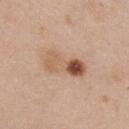Clinical impression:
The lesion was photographed on a routine skin check and not biopsied; there is no pathology result.
Image and clinical context:
A female subject aged around 45. Measured at roughly 5.5 mm in maximum diameter. The lesion is located on the chest. A lesion tile, about 15 mm wide, cut from a 3D total-body photograph. The tile uses white-light illumination.